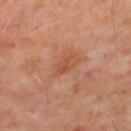biopsy_status: not biopsied; imaged during a skin examination
patient:
  sex: male
  age_approx: 60
image:
  source: total-body photography crop
  field_of_view_mm: 15
lesion_size:
  long_diameter_mm_approx: 3.0
lighting: cross-polarized
site: mid back